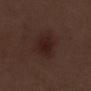Captured during whole-body skin photography for melanoma surveillance; the lesion was not biopsied.
From the right lower leg.
A 15 mm close-up tile from a total-body photography series done for melanoma screening.
The recorded lesion diameter is about 4.5 mm.
Imaged with white-light lighting.
The subject is a male aged around 70.